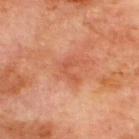Q: Was this lesion biopsied?
A: total-body-photography surveillance lesion; no biopsy
Q: Who is the patient?
A: male, approximately 70 years of age
Q: Lesion size?
A: about 3 mm
Q: What is the anatomic site?
A: the upper back
Q: What kind of image is this?
A: ~15 mm tile from a whole-body skin photo